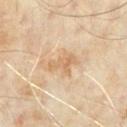Q: Was a biopsy performed?
A: total-body-photography surveillance lesion; no biopsy
Q: How was the tile lit?
A: cross-polarized illumination
Q: Who is the patient?
A: male, aged 63 to 67
Q: What kind of image is this?
A: total-body-photography crop, ~15 mm field of view
Q: Lesion size?
A: about 3.5 mm
Q: What is the anatomic site?
A: the chest